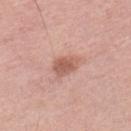follow-up = no biopsy performed (imaged during a skin exam)
lesion diameter = about 3.5 mm
subject = male, aged approximately 50
TBP lesion metrics = a mean CIELAB color near L≈58 a*≈24 b*≈28, about 11 CIELAB-L* units darker than the surrounding skin, and a normalized border contrast of about 7
body site = the left lower leg
imaging modality = total-body-photography crop, ~15 mm field of view